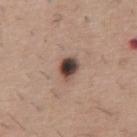biopsy status — catalogued during a skin exam; not biopsied | patient — male, in their 60s | lesion size — about 2.5 mm | illumination — white-light | image source — total-body-photography crop, ~15 mm field of view | site — the abdomen.